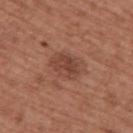| key | value |
|---|---|
| biopsy status | imaged on a skin check; not biopsied |
| automated lesion analysis | an area of roughly 7 mm², an outline eccentricity of about 0.75 (0 = round, 1 = elongated), and a symmetry-axis asymmetry near 0.2; a mean CIELAB color near L≈43 a*≈23 b*≈28 and a lesion-to-skin contrast of about 6.5 (normalized; higher = more distinct); border irregularity of about 2 on a 0–10 scale, a color-variation rating of about 3/10, and peripheral color asymmetry of about 1; an automated nevus-likeness rating near 10 out of 100 and a lesion-detection confidence of about 100/100 |
| patient | male, aged around 75 |
| body site | the upper back |
| acquisition | total-body-photography crop, ~15 mm field of view |
| lesion size | about 3.5 mm |